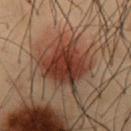The lesion was tiled from a total-body skin photograph and was not biopsied.
The subject is a male approximately 55 years of age.
Located on the abdomen.
A 15 mm crop from a total-body photograph taken for skin-cancer surveillance.